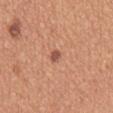Q: Is there a histopathology result?
A: total-body-photography surveillance lesion; no biopsy
Q: What is the imaging modality?
A: ~15 mm crop, total-body skin-cancer survey
Q: How was the tile lit?
A: white-light illumination
Q: Lesion size?
A: ~3 mm (longest diameter)
Q: Patient demographics?
A: male, aged 53–57
Q: What is the anatomic site?
A: the mid back
Q: Automated lesion metrics?
A: an average lesion color of about L≈58 a*≈23 b*≈31 (CIELAB) and about 7 CIELAB-L* units darker than the surrounding skin